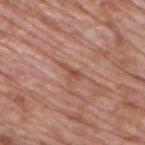Q: Was this lesion biopsied?
A: no biopsy performed (imaged during a skin exam)
Q: Who is the patient?
A: male, aged 68 to 72
Q: What is the anatomic site?
A: the back
Q: Illumination type?
A: white-light illumination
Q: What did automated image analysis measure?
A: a lesion color around L≈50 a*≈24 b*≈29 in CIELAB and a normalized border contrast of about 6.5; a border-irregularity rating of about 6.5/10 and a peripheral color-asymmetry measure near 0; an automated nevus-likeness rating near 0 out of 100
Q: Lesion size?
A: ≈3 mm
Q: What is the imaging modality?
A: 15 mm crop, total-body photography